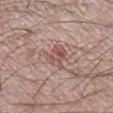The lesion was photographed on a routine skin check and not biopsied; there is no pathology result.
This image is a 15 mm lesion crop taken from a total-body photograph.
A male patient aged 58 to 62.
Located on the right lower leg.